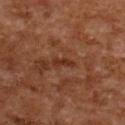lighting: cross-polarized
site: upper back
image:
  source: total-body photography crop
  field_of_view_mm: 15
patient:
  sex: female
  age_approx: 60
lesion_size:
  long_diameter_mm_approx: 3.0
automated_metrics:
  area_mm2_approx: 3.0
  eccentricity: 0.95
  shape_asymmetry: 0.35